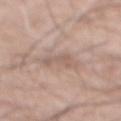notes: no biopsy performed (imaged during a skin exam)
image source: ~15 mm tile from a whole-body skin photo
automated lesion analysis: a mean CIELAB color near L≈58 a*≈16 b*≈24 and roughly 7 lightness units darker than nearby skin; border irregularity of about 6 on a 0–10 scale and a within-lesion color-variation index near 2/10; a lesion-detection confidence of about 100/100
location: the abdomen
patient: male, roughly 70 years of age
lighting: white-light